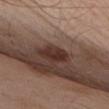follow-up: imaged on a skin check; not biopsied | patient: male, aged around 55 | site: the upper back | acquisition: total-body-photography crop, ~15 mm field of view.